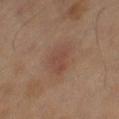Findings:
* biopsy status — no biopsy performed (imaged during a skin exam)
* body site — the right thigh
* lesion size — ~4 mm (longest diameter)
* patient — female, aged 58–62
* imaging modality — ~15 mm crop, total-body skin-cancer survey
* lighting — cross-polarized